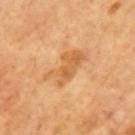Imaged during a routine full-body skin examination; the lesion was not biopsied and no histopathology is available. The recorded lesion diameter is about 5.5 mm. On the mid back. The tile uses cross-polarized illumination. A roughly 15 mm field-of-view crop from a total-body skin photograph. The subject is a male aged around 65. Automated tile analysis of the lesion measured a lesion area of about 11 mm², a shape eccentricity near 0.9, and two-axis asymmetry of about 0.35. It also reported border irregularity of about 4.5 on a 0–10 scale and a peripheral color-asymmetry measure near 1. And it measured a lesion-detection confidence of about 100/100.